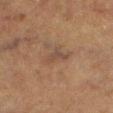This lesion was catalogued during total-body skin photography and was not selected for biopsy.
A roughly 15 mm field-of-view crop from a total-body skin photograph.
The lesion is located on the leg.
A male patient aged around 75.